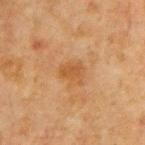Assessment:
The lesion was photographed on a routine skin check and not biopsied; there is no pathology result.
Clinical summary:
The lesion is located on the chest. Cropped from a whole-body photographic skin survey; the tile spans about 15 mm. Captured under cross-polarized illumination. The lesion's longest dimension is about 2.5 mm. An algorithmic analysis of the crop reported a footprint of about 4.5 mm² and an outline eccentricity of about 0.65 (0 = round, 1 = elongated). The software also gave a border-irregularity index near 4/10, a within-lesion color-variation index near 1/10, and a peripheral color-asymmetry measure near 0.5. A male subject, in their mid- to late 60s.